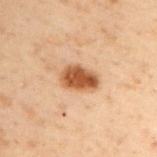Clinical summary:
A male subject, about 50 years old. The lesion is located on the upper back. A 15 mm close-up tile from a total-body photography series done for melanoma screening. Approximately 4 mm at its widest. Automated tile analysis of the lesion measured a footprint of about 8.5 mm² and an outline eccentricity of about 0.8 (0 = round, 1 = elongated). Imaged with cross-polarized lighting.